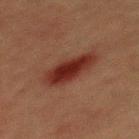{
  "biopsy_status": "not biopsied; imaged during a skin examination",
  "lesion_size": {
    "long_diameter_mm_approx": 5.5
  },
  "image": {
    "source": "total-body photography crop",
    "field_of_view_mm": 15
  },
  "patient": {
    "sex": "male",
    "age_approx": 40
  },
  "automated_metrics": {
    "eccentricity": 0.85,
    "shape_asymmetry": 0.2,
    "cielab_L": 25,
    "cielab_a": 23,
    "cielab_b": 23,
    "vs_skin_darker_L": 10.0,
    "vs_skin_contrast_norm": 10.5,
    "nevus_likeness_0_100": 100,
    "lesion_detection_confidence_0_100": 100
  },
  "site": "mid back",
  "lighting": "cross-polarized"
}